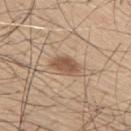<lesion>
  <biopsy_status>not biopsied; imaged during a skin examination</biopsy_status>
  <patient>
    <sex>male</sex>
    <age_approx>55</age_approx>
  </patient>
  <image>
    <source>total-body photography crop</source>
    <field_of_view_mm>15</field_of_view_mm>
  </image>
  <site>right upper arm</site>
  <lighting>white-light</lighting>
  <lesion_size>
    <long_diameter_mm_approx>3.5</long_diameter_mm_approx>
  </lesion_size>
</lesion>